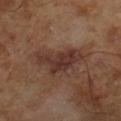Imaged during a routine full-body skin examination; the lesion was not biopsied and no histopathology is available. This is a cross-polarized tile. A male subject in their mid- to late 60s. This image is a 15 mm lesion crop taken from a total-body photograph. The total-body-photography lesion software estimated a border-irregularity rating of about 6.5/10.